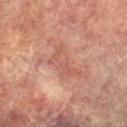* notes — no biopsy performed (imaged during a skin exam)
* image — total-body-photography crop, ~15 mm field of view
* image-analysis metrics — an area of roughly 8.5 mm² and an outline eccentricity of about 0.85 (0 = round, 1 = elongated); a color-variation rating of about 3/10; a nevus-likeness score of about 0/100 and lesion-presence confidence of about 90/100
* tile lighting — cross-polarized illumination
* body site — the right lower leg
* size — about 4.5 mm
* subject — male, roughly 75 years of age